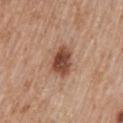Image and clinical context: Measured at roughly 4 mm in maximum diameter. A 15 mm crop from a total-body photograph taken for skin-cancer surveillance. The subject is a male aged 58 to 62. Located on the mid back. The total-body-photography lesion software estimated a mean CIELAB color near L≈47 a*≈22 b*≈29, a lesion–skin lightness drop of about 15, and a normalized lesion–skin contrast near 10.5. It also reported a color-variation rating of about 4.5/10 and peripheral color asymmetry of about 1.5. This is a white-light tile.